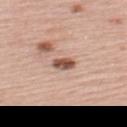<record>
  <biopsy_status>not biopsied; imaged during a skin examination</biopsy_status>
  <image>
    <source>total-body photography crop</source>
    <field_of_view_mm>15</field_of_view_mm>
  </image>
  <lighting>white-light</lighting>
  <patient>
    <sex>male</sex>
    <age_approx>55</age_approx>
  </patient>
  <site>upper back</site>
  <automated_metrics>
    <area_mm2_approx>4.0</area_mm2_approx>
    <eccentricity>0.8</eccentricity>
    <shape_asymmetry>0.2</shape_asymmetry>
    <vs_skin_darker_L>16.0</vs_skin_darker_L>
    <vs_skin_contrast_norm>10.5</vs_skin_contrast_norm>
    <color_variation_0_10>4.0</color_variation_0_10>
    <peripheral_color_asymmetry>1.5</peripheral_color_asymmetry>
    <nevus_likeness_0_100>80</nevus_likeness_0_100>
  </automated_metrics>
</record>